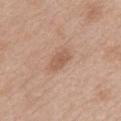Q: Was a biopsy performed?
A: total-body-photography surveillance lesion; no biopsy
Q: What is the imaging modality?
A: ~15 mm crop, total-body skin-cancer survey
Q: What is the anatomic site?
A: the mid back
Q: Patient demographics?
A: female, aged 48 to 52
Q: Illumination type?
A: white-light